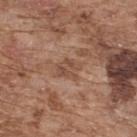Part of a total-body skin-imaging series; this lesion was reviewed on a skin check and was not flagged for biopsy.
The lesion is on the upper back.
A male patient aged 73 to 77.
This is a white-light tile.
A 15 mm crop from a total-body photograph taken for skin-cancer surveillance.
Automated tile analysis of the lesion measured a border-irregularity index near 3.5/10, a within-lesion color-variation index near 3.5/10, and radial color variation of about 1. The analysis additionally found a nevus-likeness score of about 0/100 and a detector confidence of about 100 out of 100 that the crop contains a lesion.